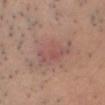tile lighting — white-light illumination | body site — the head or neck | patient — male, roughly 40 years of age | acquisition — ~15 mm tile from a whole-body skin photo | diameter — ~6 mm (longest diameter).